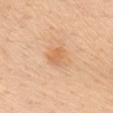Clinical impression: Recorded during total-body skin imaging; not selected for excision or biopsy. Image and clinical context: Automated tile analysis of the lesion measured roughly 8 lightness units darker than nearby skin and a normalized lesion–skin contrast near 5.5. It also reported a classifier nevus-likeness of about 10/100 and lesion-presence confidence of about 100/100. Captured under white-light illumination. A female subject aged 43 to 47. From the chest. This image is a 15 mm lesion crop taken from a total-body photograph. The recorded lesion diameter is about 2.5 mm.